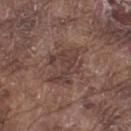Impression: Recorded during total-body skin imaging; not selected for excision or biopsy. Acquisition and patient details: This image is a 15 mm lesion crop taken from a total-body photograph. The recorded lesion diameter is about 5.5 mm. The lesion is located on the left thigh. A male subject, in their mid-70s.